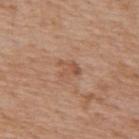Located on the back. A male patient in their 60s. Approximately 2.5 mm at its widest. Captured under white-light illumination. A lesion tile, about 15 mm wide, cut from a 3D total-body photograph.